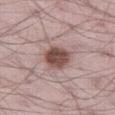A male subject, about 70 years old. A 15 mm close-up tile from a total-body photography series done for melanoma screening. Longest diameter approximately 3.5 mm. The lesion is on the right thigh. This is a white-light tile.